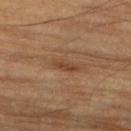Findings:
– lesion size — ≈3.5 mm
– site — the right thigh
– image source — ~15 mm tile from a whole-body skin photo
– subject — male, aged approximately 85 or older
– lighting — cross-polarized